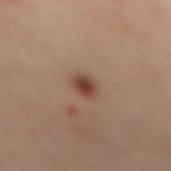Captured during whole-body skin photography for melanoma surveillance; the lesion was not biopsied. The lesion's longest dimension is about 3 mm. A female subject approximately 55 years of age. The lesion is located on the mid back. The tile uses cross-polarized illumination. Automated tile analysis of the lesion measured a lesion color around L≈36 a*≈17 b*≈23 in CIELAB, a lesion–skin lightness drop of about 10, and a normalized lesion–skin contrast near 9. The analysis additionally found a nevus-likeness score of about 100/100. A region of skin cropped from a whole-body photographic capture, roughly 15 mm wide.